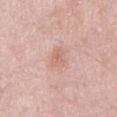Captured during whole-body skin photography for melanoma surveillance; the lesion was not biopsied. The recorded lesion diameter is about 2.5 mm. A male patient aged 53–57. Cropped from a whole-body photographic skin survey; the tile spans about 15 mm. The lesion is located on the abdomen. The tile uses white-light illumination.